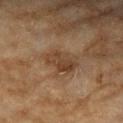Q: Is there a histopathology result?
A: imaged on a skin check; not biopsied
Q: Who is the patient?
A: female, roughly 60 years of age
Q: Automated lesion metrics?
A: a lesion color around L≈38 a*≈16 b*≈29 in CIELAB, about 8 CIELAB-L* units darker than the surrounding skin, and a normalized lesion–skin contrast near 7; a color-variation rating of about 4/10 and peripheral color asymmetry of about 1.5; a classifier nevus-likeness of about 0/100 and a lesion-detection confidence of about 100/100
Q: What is the lesion's diameter?
A: about 4.5 mm
Q: What is the imaging modality?
A: 15 mm crop, total-body photography
Q: What is the anatomic site?
A: the right upper arm
Q: Illumination type?
A: cross-polarized illumination